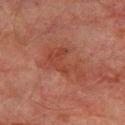- follow-up · imaged on a skin check; not biopsied
- size · ≈5.5 mm
- patient · male, aged 73–77
- location · the right lower leg
- lighting · cross-polarized illumination
- image source · total-body-photography crop, ~15 mm field of view